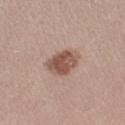Image and clinical context: A close-up tile cropped from a whole-body skin photograph, about 15 mm across. A female subject, in their 20s. The lesion is located on the right thigh. Automated tile analysis of the lesion measured a footprint of about 9.5 mm², an eccentricity of roughly 0.55, and two-axis asymmetry of about 0.2. And it measured a border-irregularity rating of about 2/10, a within-lesion color-variation index near 4.5/10, and radial color variation of about 1.5. Imaged with white-light lighting. The lesion's longest dimension is about 3.5 mm.